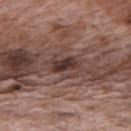The lesion was photographed on a routine skin check and not biopsied; there is no pathology result.
From the mid back.
Automated tile analysis of the lesion measured a mean CIELAB color near L≈38 a*≈18 b*≈20, roughly 10 lightness units darker than nearby skin, and a lesion-to-skin contrast of about 8.5 (normalized; higher = more distinct).
The subject is a male aged 68 to 72.
Cropped from a whole-body photographic skin survey; the tile spans about 15 mm.
Imaged with white-light lighting.
Measured at roughly 4.5 mm in maximum diameter.